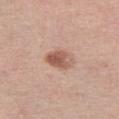– follow-up — catalogued during a skin exam; not biopsied
– TBP lesion metrics — a border-irregularity rating of about 1.5/10, a color-variation rating of about 6/10, and radial color variation of about 2.5
– image source — ~15 mm crop, total-body skin-cancer survey
– diameter — about 3.5 mm
– illumination — white-light illumination
– anatomic site — the left thigh
– patient — female, aged 48 to 52